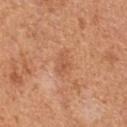Findings:
* notes: total-body-photography surveillance lesion; no biopsy
* automated metrics: internal color variation of about 2 on a 0–10 scale and radial color variation of about 1
* subject: male, aged around 55
* location: the chest
* image: 15 mm crop, total-body photography
* diameter: ≈3 mm
* illumination: white-light